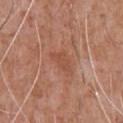workup: total-body-photography surveillance lesion; no biopsy
TBP lesion metrics: an average lesion color of about L≈50 a*≈25 b*≈32 (CIELAB), a lesion–skin lightness drop of about 6, and a normalized border contrast of about 5; an automated nevus-likeness rating near 0 out of 100
tile lighting: white-light illumination
size: ≈3 mm
patient: male, aged 58–62
acquisition: ~15 mm crop, total-body skin-cancer survey
anatomic site: the upper back